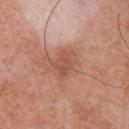Q: Was a biopsy performed?
A: no biopsy performed (imaged during a skin exam)
Q: What is the lesion's diameter?
A: ≈3 mm
Q: Automated lesion metrics?
A: an area of roughly 3.5 mm² and a shape-asymmetry score of about 0.35 (0 = symmetric); a lesion color around L≈52 a*≈25 b*≈29 in CIELAB and a normalized border contrast of about 5.5; a within-lesion color-variation index near 1.5/10; a nevus-likeness score of about 10/100 and lesion-presence confidence of about 100/100
Q: How was the tile lit?
A: white-light
Q: Who is the patient?
A: male, aged 68–72
Q: How was this image acquired?
A: ~15 mm tile from a whole-body skin photo
Q: Where on the body is the lesion?
A: the chest